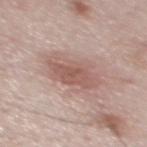workup = no biopsy performed (imaged during a skin exam) | subject = male, roughly 45 years of age | anatomic site = the mid back | image source = total-body-photography crop, ~15 mm field of view | lighting = white-light illumination.